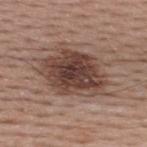Clinical summary: The patient is a male about 40 years old. Measured at roughly 9 mm in maximum diameter. The lesion is on the upper back. The total-body-photography lesion software estimated an area of roughly 30 mm² and an outline eccentricity of about 0.75 (0 = round, 1 = elongated). It also reported a lesion color around L≈43 a*≈18 b*≈23 in CIELAB, roughly 14 lightness units darker than nearby skin, and a normalized lesion–skin contrast near 10.5. The software also gave a nevus-likeness score of about 85/100 and a detector confidence of about 100 out of 100 that the crop contains a lesion. A lesion tile, about 15 mm wide, cut from a 3D total-body photograph. The tile uses white-light illumination.